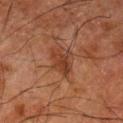follow-up=total-body-photography surveillance lesion; no biopsy
illumination=cross-polarized illumination
subject=male, aged 78–82
image source=15 mm crop, total-body photography
size=about 4 mm
site=the left thigh